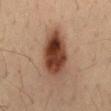Imaged during a routine full-body skin examination; the lesion was not biopsied and no histopathology is available. A lesion tile, about 15 mm wide, cut from a 3D total-body photograph. A male subject aged approximately 35. Located on the back. Captured under cross-polarized illumination. Longest diameter approximately 7 mm. The lesion-visualizer software estimated a classifier nevus-likeness of about 100/100.